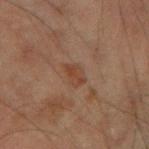Captured during whole-body skin photography for melanoma surveillance; the lesion was not biopsied. Located on the left forearm. A male patient roughly 60 years of age. The recorded lesion diameter is about 3 mm. This is a cross-polarized tile. A roughly 15 mm field-of-view crop from a total-body skin photograph.